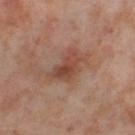biopsy status — no biopsy performed (imaged during a skin exam) | patient — female, approximately 55 years of age | location — the right thigh | lighting — cross-polarized illumination | diameter — ≈4 mm | acquisition — 15 mm crop, total-body photography | image-analysis metrics — an average lesion color of about L≈46 a*≈22 b*≈29 (CIELAB), roughly 9 lightness units darker than nearby skin, and a normalized border contrast of about 7; a border-irregularity rating of about 4.5/10 and a within-lesion color-variation index near 5/10; lesion-presence confidence of about 100/100.